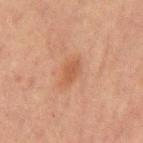Impression: The lesion was tiled from a total-body skin photograph and was not biopsied. Background: This is a cross-polarized tile. A male patient aged approximately 65. Longest diameter approximately 3 mm. From the abdomen. A 15 mm crop from a total-body photograph taken for skin-cancer surveillance. Automated image analysis of the tile measured an area of roughly 4 mm² and an eccentricity of roughly 0.85. The software also gave a mean CIELAB color near L≈44 a*≈20 b*≈29, about 7 CIELAB-L* units darker than the surrounding skin, and a lesion-to-skin contrast of about 6 (normalized; higher = more distinct). And it measured an automated nevus-likeness rating near 20 out of 100.